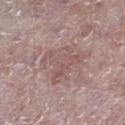Cropped from a total-body skin-imaging series; the visible field is about 15 mm. An algorithmic analysis of the crop reported roughly 7 lightness units darker than nearby skin and a normalized lesion–skin contrast near 5. And it measured a border-irregularity index near 7.5/10, internal color variation of about 3.5 on a 0–10 scale, and radial color variation of about 1. The software also gave an automated nevus-likeness rating near 0 out of 100 and a detector confidence of about 95 out of 100 that the crop contains a lesion. A male subject, about 75 years old. The tile uses white-light illumination. The lesion is located on the right lower leg. The lesion's longest dimension is about 5 mm.